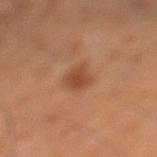workup = imaged on a skin check; not biopsied
imaging modality = total-body-photography crop, ~15 mm field of view
subject = male, aged around 60
anatomic site = the left lower leg